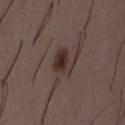Captured during whole-body skin photography for melanoma surveillance; the lesion was not biopsied. The patient is a male roughly 50 years of age. From the abdomen. A roughly 15 mm field-of-view crop from a total-body skin photograph.